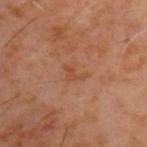Image and clinical context: The patient is a male aged approximately 60. On the back. A close-up tile cropped from a whole-body skin photograph, about 15 mm across. Captured under cross-polarized illumination. About 2.5 mm across.